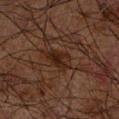Clinical impression: No biopsy was performed on this lesion — it was imaged during a full skin examination and was not determined to be concerning. Clinical summary: The recorded lesion diameter is about 3.5 mm. An algorithmic analysis of the crop reported a footprint of about 6 mm² and two-axis asymmetry of about 0.25. Captured under cross-polarized illumination. A 15 mm close-up extracted from a 3D total-body photography capture. A male patient approximately 70 years of age. The lesion is on the right forearm.